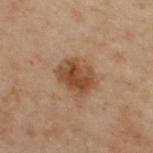No biopsy was performed on this lesion — it was imaged during a full skin examination and was not determined to be concerning.